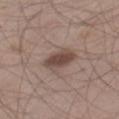This lesion was catalogued during total-body skin photography and was not selected for biopsy. This is a white-light tile. A lesion tile, about 15 mm wide, cut from a 3D total-body photograph. On the right thigh. The lesion's longest dimension is about 4 mm. A male subject, roughly 60 years of age.